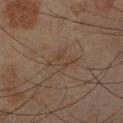The lesion was photographed on a routine skin check and not biopsied; there is no pathology result.
On the right lower leg.
Approximately 3.5 mm at its widest.
The patient is a male roughly 45 years of age.
A 15 mm crop from a total-body photograph taken for skin-cancer surveillance.